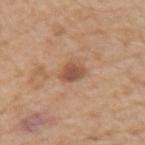biopsy status: catalogued during a skin exam; not biopsied | location: the right upper arm | subject: male, roughly 70 years of age | acquisition: ~15 mm tile from a whole-body skin photo | lesion size: about 3 mm | automated lesion analysis: a lesion area of about 5 mm², an eccentricity of roughly 0.65, and a symmetry-axis asymmetry near 0.15; a border-irregularity rating of about 1.5/10, internal color variation of about 3 on a 0–10 scale, and peripheral color asymmetry of about 1.